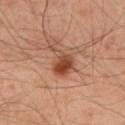Impression:
No biopsy was performed on this lesion — it was imaged during a full skin examination and was not determined to be concerning.
Acquisition and patient details:
About 4 mm across. The lesion is located on the mid back. Cropped from a whole-body photographic skin survey; the tile spans about 15 mm. Automated tile analysis of the lesion measured a lesion color around L≈44 a*≈24 b*≈31 in CIELAB, about 11 CIELAB-L* units darker than the surrounding skin, and a lesion-to-skin contrast of about 9 (normalized; higher = more distinct). And it measured a classifier nevus-likeness of about 95/100 and lesion-presence confidence of about 100/100. This is a cross-polarized tile. The patient is a male aged approximately 45.